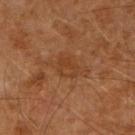Impression:
This lesion was catalogued during total-body skin photography and was not selected for biopsy.
Background:
The tile uses cross-polarized illumination. The recorded lesion diameter is about 3 mm. Cropped from a whole-body photographic skin survey; the tile spans about 15 mm. Located on the right upper arm. The lesion-visualizer software estimated a footprint of about 4.5 mm², a shape eccentricity near 0.65, and a shape-asymmetry score of about 0.25 (0 = symmetric). The subject is a male aged approximately 60.